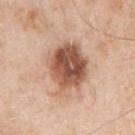Impression:
Captured during whole-body skin photography for melanoma surveillance; the lesion was not biopsied.
Context:
Approximately 6 mm at its widest. A male patient, in their mid-50s. The lesion is located on the left upper arm. The lesion-visualizer software estimated a footprint of about 22 mm², a shape eccentricity near 0.65, and two-axis asymmetry of about 0.2. And it measured a normalized lesion–skin contrast near 11. The analysis additionally found a border-irregularity rating of about 2.5/10. The tile uses white-light illumination. A 15 mm crop from a total-body photograph taken for skin-cancer surveillance.